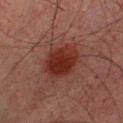| feature | finding |
|---|---|
| location | the chest |
| size | ~4.5 mm (longest diameter) |
| imaging modality | ~15 mm crop, total-body skin-cancer survey |
| automated metrics | a nevus-likeness score of about 100/100 |
| patient | male, about 30 years old |
| lighting | cross-polarized illumination |